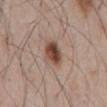Impression:
Recorded during total-body skin imaging; not selected for excision or biopsy.
Clinical summary:
This is a white-light tile. On the abdomen. The lesion's longest dimension is about 3 mm. A 15 mm close-up tile from a total-body photography series done for melanoma screening. A male subject, aged around 55.